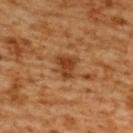Q: Was a biopsy performed?
A: catalogued during a skin exam; not biopsied
Q: Patient demographics?
A: female, roughly 55 years of age
Q: How was this image acquired?
A: 15 mm crop, total-body photography
Q: Where on the body is the lesion?
A: the upper back